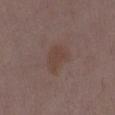Image and clinical context: A 15 mm close-up extracted from a 3D total-body photography capture. The recorded lesion diameter is about 4 mm. This is a white-light tile. The lesion is on the back. A female subject, aged 33 to 37.